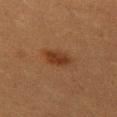Recorded during total-body skin imaging; not selected for excision or biopsy. The lesion is located on the left thigh. A region of skin cropped from a whole-body photographic capture, roughly 15 mm wide. A female subject aged 38–42.